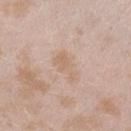<tbp_lesion>
  <biopsy_status>not biopsied; imaged during a skin examination</biopsy_status>
  <patient>
    <sex>female</sex>
    <age_approx>25</age_approx>
  </patient>
  <image>
    <source>total-body photography crop</source>
    <field_of_view_mm>15</field_of_view_mm>
  </image>
  <lighting>white-light</lighting>
  <site>right forearm</site>
  <automated_metrics>
    <area_mm2_approx>5.5</area_mm2_approx>
    <eccentricity>0.9</eccentricity>
    <shape_asymmetry>0.5</shape_asymmetry>
  </automated_metrics>
</tbp_lesion>